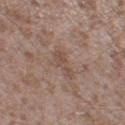Q: Is there a histopathology result?
A: total-body-photography surveillance lesion; no biopsy
Q: Lesion size?
A: about 3.5 mm
Q: Automated lesion metrics?
A: a footprint of about 3.5 mm² and two-axis asymmetry of about 0.5; border irregularity of about 6 on a 0–10 scale and internal color variation of about 0.5 on a 0–10 scale
Q: Illumination type?
A: white-light
Q: How was this image acquired?
A: ~15 mm tile from a whole-body skin photo
Q: What are the patient's age and sex?
A: male, aged approximately 45
Q: Lesion location?
A: the left thigh